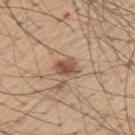• workup: no biopsy performed (imaged during a skin exam)
• patient: male, roughly 55 years of age
• lesion diameter: about 3 mm
• TBP lesion metrics: a footprint of about 5 mm² and a shape-asymmetry score of about 0.25 (0 = symmetric); a classifier nevus-likeness of about 80/100 and a lesion-detection confidence of about 100/100
• location: the left upper arm
• illumination: white-light illumination
• image source: 15 mm crop, total-body photography